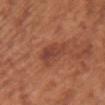Impression:
Imaged during a routine full-body skin examination; the lesion was not biopsied and no histopathology is available.
Context:
This image is a 15 mm lesion crop taken from a total-body photograph. The lesion-visualizer software estimated an eccentricity of roughly 0.85. Located on the chest. The recorded lesion diameter is about 4 mm. A female subject, roughly 65 years of age.